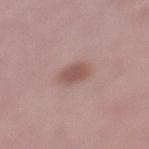Part of a total-body skin-imaging series; this lesion was reviewed on a skin check and was not flagged for biopsy.
A female patient aged around 40.
Located on the left lower leg.
The lesion-visualizer software estimated an eccentricity of roughly 0.6 and a symmetry-axis asymmetry near 0.15. It also reported a nevus-likeness score of about 95/100.
Measured at roughly 3 mm in maximum diameter.
A 15 mm close-up extracted from a 3D total-body photography capture.
Captured under white-light illumination.